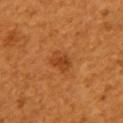lighting: cross-polarized
patient:
  sex: female
  age_approx: 55
site: right upper arm
image:
  source: total-body photography crop
  field_of_view_mm: 15
automated_metrics:
  nevus_likeness_0_100: 85
  lesion_detection_confidence_0_100: 100
lesion_size:
  long_diameter_mm_approx: 3.0